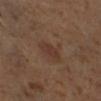The lesion was photographed on a routine skin check and not biopsied; there is no pathology result. From the right lower leg. A roughly 15 mm field-of-view crop from a total-body skin photograph. The recorded lesion diameter is about 3 mm. Captured under cross-polarized illumination. The subject is a female aged 48–52.